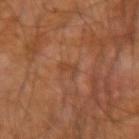Q: Was this lesion biopsied?
A: no biopsy performed (imaged during a skin exam)
Q: Lesion location?
A: the right forearm
Q: Who is the patient?
A: male, roughly 60 years of age
Q: What lighting was used for the tile?
A: cross-polarized
Q: What is the imaging modality?
A: 15 mm crop, total-body photography
Q: What did automated image analysis measure?
A: a lesion area of about 2.5 mm², an outline eccentricity of about 0.9 (0 = round, 1 = elongated), and a shape-asymmetry score of about 0.45 (0 = symmetric); a border-irregularity index near 5/10 and internal color variation of about 0 on a 0–10 scale; an automated nevus-likeness rating near 0 out of 100 and a detector confidence of about 100 out of 100 that the crop contains a lesion